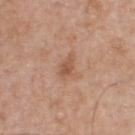follow-up: no biopsy performed (imaged during a skin exam)
image-analysis metrics: a border-irregularity index near 3/10, internal color variation of about 2 on a 0–10 scale, and peripheral color asymmetry of about 0.5
subject: male, aged around 50
location: the front of the torso
imaging modality: total-body-photography crop, ~15 mm field of view
size: ~3 mm (longest diameter)